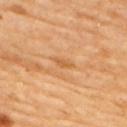workup: catalogued during a skin exam; not biopsied | acquisition: ~15 mm crop, total-body skin-cancer survey | site: the arm | subject: female, aged 68–72 | diameter: about 2.5 mm | tile lighting: cross-polarized | automated lesion analysis: border irregularity of about 3.5 on a 0–10 scale and peripheral color asymmetry of about 0.